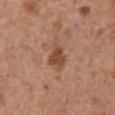{
  "biopsy_status": "not biopsied; imaged during a skin examination",
  "lighting": "white-light",
  "lesion_size": {
    "long_diameter_mm_approx": 3.5
  },
  "automated_metrics": {
    "area_mm2_approx": 5.0,
    "eccentricity": 0.7,
    "shape_asymmetry": 0.35,
    "border_irregularity_0_10": 3.0,
    "peripheral_color_asymmetry": 0.5
  },
  "image": {
    "source": "total-body photography crop",
    "field_of_view_mm": 15
  },
  "patient": {
    "sex": "male",
    "age_approx": 65
  },
  "site": "front of the torso"
}